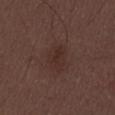Q: Was this lesion biopsied?
A: total-body-photography surveillance lesion; no biopsy
Q: Who is the patient?
A: male, in their 30s
Q: Where on the body is the lesion?
A: the abdomen
Q: How was this image acquired?
A: ~15 mm crop, total-body skin-cancer survey